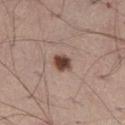{
  "lighting": "white-light",
  "image": {
    "source": "total-body photography crop",
    "field_of_view_mm": 15
  },
  "automated_metrics": {
    "cielab_L": 45,
    "cielab_a": 19,
    "cielab_b": 24,
    "vs_skin_darker_L": 16.0,
    "border_irregularity_0_10": 1.5,
    "color_variation_0_10": 3.5
  },
  "lesion_size": {
    "long_diameter_mm_approx": 2.5
  },
  "patient": {
    "sex": "male",
    "age_approx": 55
  },
  "site": "left thigh"
}